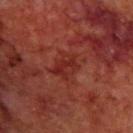Captured during whole-body skin photography for melanoma surveillance; the lesion was not biopsied. A male subject, aged around 70. A 15 mm crop from a total-body photograph taken for skin-cancer surveillance. This is a cross-polarized tile. The lesion-visualizer software estimated a footprint of about 8.5 mm² and a shape eccentricity near 0.5. The software also gave a border-irregularity rating of about 4/10, a color-variation rating of about 4/10, and radial color variation of about 1.5. The software also gave a classifier nevus-likeness of about 0/100 and a lesion-detection confidence of about 90/100. The lesion is on the upper back. Approximately 3.5 mm at its widest.